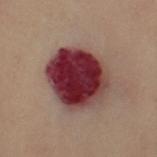Assessment: Imaged during a routine full-body skin examination; the lesion was not biopsied and no histopathology is available. Background: A 15 mm close-up tile from a total-body photography series done for melanoma screening. This is a cross-polarized tile. About 6.5 mm across. Located on the left leg. A female subject, in their 60s.